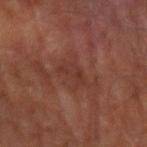– workup · catalogued during a skin exam; not biopsied
– tile lighting · cross-polarized illumination
– site · the arm
– patient · male, about 70 years old
– automated metrics · a lesion color around L≈27 a*≈19 b*≈21 in CIELAB and roughly 5 lightness units darker than nearby skin; a color-variation rating of about 0/10 and radial color variation of about 0; a nevus-likeness score of about 0/100 and a lesion-detection confidence of about 90/100
– diameter · ≈3 mm
– acquisition · total-body-photography crop, ~15 mm field of view